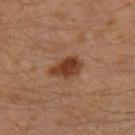This lesion was catalogued during total-body skin photography and was not selected for biopsy.
Automated image analysis of the tile measured border irregularity of about 3 on a 0–10 scale, internal color variation of about 3.5 on a 0–10 scale, and radial color variation of about 1.5. And it measured a classifier nevus-likeness of about 95/100.
This is a cross-polarized tile.
A male patient, in their 30s.
The lesion's longest dimension is about 4 mm.
A lesion tile, about 15 mm wide, cut from a 3D total-body photograph.
From the left leg.